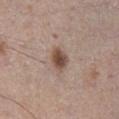biopsy status: no biopsy performed (imaged during a skin exam) | acquisition: ~15 mm crop, total-body skin-cancer survey | tile lighting: white-light | anatomic site: the right lower leg | image-analysis metrics: a footprint of about 5.5 mm², an outline eccentricity of about 0.65 (0 = round, 1 = elongated), and a shape-asymmetry score of about 0.1 (0 = symmetric); roughly 13 lightness units darker than nearby skin and a normalized border contrast of about 9.5; a nevus-likeness score of about 95/100 and a lesion-detection confidence of about 100/100 | patient: male, aged around 55.